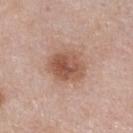Q: Was a biopsy performed?
A: catalogued during a skin exam; not biopsied
Q: Patient demographics?
A: male, aged around 55
Q: Where on the body is the lesion?
A: the chest
Q: How was this image acquired?
A: 15 mm crop, total-body photography